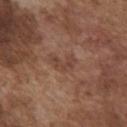Findings:
– biopsy status — imaged on a skin check; not biopsied
– subject — male, about 75 years old
– automated metrics — a color-variation rating of about 1.5/10 and peripheral color asymmetry of about 0.5; an automated nevus-likeness rating near 0 out of 100
– acquisition — ~15 mm crop, total-body skin-cancer survey
– body site — the chest
– lighting — white-light
– size — ≈3 mm